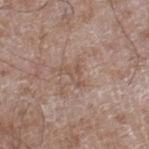Recorded during total-body skin imaging; not selected for excision or biopsy.
Automated tile analysis of the lesion measured an average lesion color of about L≈52 a*≈17 b*≈26 (CIELAB) and a lesion-to-skin contrast of about 5 (normalized; higher = more distinct).
Cropped from a whole-body photographic skin survey; the tile spans about 15 mm.
About 3 mm across.
A male patient, aged approximately 60.
Captured under white-light illumination.
The lesion is on the right lower leg.